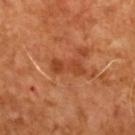Part of a total-body skin-imaging series; this lesion was reviewed on a skin check and was not flagged for biopsy. The tile uses cross-polarized illumination. The lesion-visualizer software estimated a lesion–skin lightness drop of about 9. The software also gave border irregularity of about 4.5 on a 0–10 scale, internal color variation of about 2.5 on a 0–10 scale, and peripheral color asymmetry of about 0.5. The software also gave a lesion-detection confidence of about 100/100. The subject is a male roughly 65 years of age. Cropped from a total-body skin-imaging series; the visible field is about 15 mm.